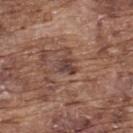follow-up: catalogued during a skin exam; not biopsied
subject: male, in their mid-70s
lesion diameter: about 2.5 mm
imaging modality: ~15 mm crop, total-body skin-cancer survey
illumination: white-light illumination
anatomic site: the upper back